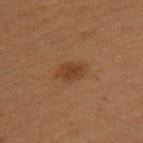Q: Was this lesion biopsied?
A: imaged on a skin check; not biopsied
Q: Automated lesion metrics?
A: a lesion area of about 5 mm² and two-axis asymmetry of about 0.2; a mean CIELAB color near L≈34 a*≈19 b*≈29, roughly 7 lightness units darker than nearby skin, and a lesion-to-skin contrast of about 7 (normalized; higher = more distinct); a border-irregularity rating of about 2/10 and internal color variation of about 2 on a 0–10 scale
Q: How was this image acquired?
A: total-body-photography crop, ~15 mm field of view
Q: How was the tile lit?
A: cross-polarized
Q: Who is the patient?
A: female, about 50 years old
Q: How large is the lesion?
A: ~3.5 mm (longest diameter)
Q: What is the anatomic site?
A: the left upper arm